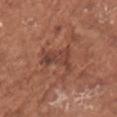Part of a total-body skin-imaging series; this lesion was reviewed on a skin check and was not flagged for biopsy. A female subject, roughly 75 years of age. A close-up tile cropped from a whole-body skin photograph, about 15 mm across. Located on the back.